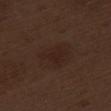Assessment: Captured during whole-body skin photography for melanoma surveillance; the lesion was not biopsied. Context: This is a white-light tile. Automated image analysis of the tile measured a footprint of about 14 mm². And it measured a lesion-detection confidence of about 100/100. Approximately 5 mm at its widest. A male patient, in their 70s. A region of skin cropped from a whole-body photographic capture, roughly 15 mm wide. From the left thigh.